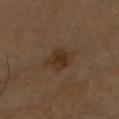The tile uses cross-polarized illumination.
A female subject, aged 68–72.
From the right forearm.
A roughly 15 mm field-of-view crop from a total-body skin photograph.